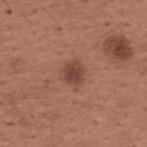Clinical impression: Captured during whole-body skin photography for melanoma surveillance; the lesion was not biopsied. Background: A male patient, aged 63–67. The lesion is located on the upper back. This is a white-light tile. Automated image analysis of the tile measured a lesion area of about 5.5 mm², an eccentricity of roughly 0.5, and a symmetry-axis asymmetry near 0.3. The analysis additionally found radial color variation of about 1. A roughly 15 mm field-of-view crop from a total-body skin photograph.